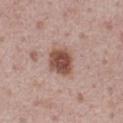follow-up: total-body-photography surveillance lesion; no biopsy
body site: the chest
acquisition: 15 mm crop, total-body photography
patient: male, about 75 years old
lighting: white-light illumination
size: ≈3.5 mm